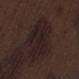Clinical impression:
Part of a total-body skin-imaging series; this lesion was reviewed on a skin check and was not flagged for biopsy.
Background:
Located on the right thigh. A 15 mm crop from a total-body photograph taken for skin-cancer surveillance. A male subject approximately 70 years of age.